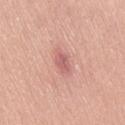– follow-up · no biopsy performed (imaged during a skin exam)
– image source · ~15 mm crop, total-body skin-cancer survey
– tile lighting · white-light illumination
– size · ≈2.5 mm
– subject · male, aged 53 to 57
– site · the right thigh